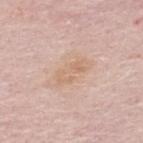Clinical impression:
Captured during whole-body skin photography for melanoma surveillance; the lesion was not biopsied.
Background:
The lesion is on the upper back. A 15 mm crop from a total-body photograph taken for skin-cancer surveillance. A male patient roughly 65 years of age. The lesion-visualizer software estimated a lesion–skin lightness drop of about 6 and a normalized border contrast of about 5.5. And it measured a border-irregularity index near 5/10, a color-variation rating of about 3/10, and peripheral color asymmetry of about 1. The analysis additionally found a nevus-likeness score of about 0/100.